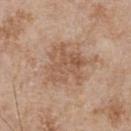subject — male, in their mid-60s
lighting — white-light illumination
location — the chest
acquisition — total-body-photography crop, ~15 mm field of view
size — ≈4.5 mm
image-analysis metrics — an area of roughly 16 mm² and a shape eccentricity near 0.35; a lesion color around L≈55 a*≈19 b*≈31 in CIELAB, about 8 CIELAB-L* units darker than the surrounding skin, and a lesion-to-skin contrast of about 6 (normalized; higher = more distinct); border irregularity of about 5 on a 0–10 scale and a within-lesion color-variation index near 4.5/10; an automated nevus-likeness rating near 0 out of 100